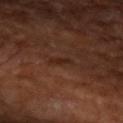{"biopsy_status": "not biopsied; imaged during a skin examination", "lighting": "cross-polarized", "automated_metrics": {"cielab_L": 24, "cielab_a": 20, "cielab_b": 24, "vs_skin_darker_L": 5.0, "border_irregularity_0_10": 4.0, "nevus_likeness_0_100": 0, "lesion_detection_confidence_0_100": 100}, "site": "left upper arm", "image": {"source": "total-body photography crop", "field_of_view_mm": 15}, "lesion_size": {"long_diameter_mm_approx": 2.5}, "patient": {"age_approx": 65}}